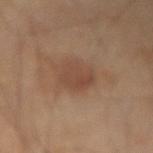Captured during whole-body skin photography for melanoma surveillance; the lesion was not biopsied. Measured at roughly 4 mm in maximum diameter. A region of skin cropped from a whole-body photographic capture, roughly 15 mm wide. From the left forearm. A male subject, aged 68 to 72.